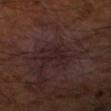Part of a total-body skin-imaging series; this lesion was reviewed on a skin check and was not flagged for biopsy. The subject is a male about 65 years old. Imaged with cross-polarized lighting. A close-up tile cropped from a whole-body skin photograph, about 15 mm across.